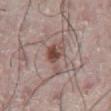notes: imaged on a skin check; not biopsied
image: ~15 mm tile from a whole-body skin photo
subject: male, aged 73 to 77
location: the abdomen
TBP lesion metrics: a mean CIELAB color near L≈49 a*≈19 b*≈22, roughly 11 lightness units darker than nearby skin, and a normalized border contrast of about 8.5; border irregularity of about 7 on a 0–10 scale, a color-variation rating of about 8/10, and peripheral color asymmetry of about 3; an automated nevus-likeness rating near 85 out of 100 and a detector confidence of about 100 out of 100 that the crop contains a lesion